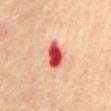  image:
    source: total-body photography crop
    field_of_view_mm: 15
  patient:
    sex: female
    age_approx: 70
  site: mid back